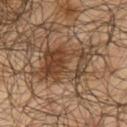Part of a total-body skin-imaging series; this lesion was reviewed on a skin check and was not flagged for biopsy. The lesion is located on the arm. A male subject about 65 years old. Measured at roughly 8 mm in maximum diameter. The total-body-photography lesion software estimated an area of roughly 21 mm², an outline eccentricity of about 0.85 (0 = round, 1 = elongated), and a shape-asymmetry score of about 0.4 (0 = symmetric). The analysis additionally found a border-irregularity rating of about 6.5/10 and internal color variation of about 8.5 on a 0–10 scale. The software also gave an automated nevus-likeness rating near 40 out of 100 and a lesion-detection confidence of about 55/100. Imaged with cross-polarized lighting. A lesion tile, about 15 mm wide, cut from a 3D total-body photograph.